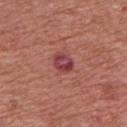Assessment:
Recorded during total-body skin imaging; not selected for excision or biopsy.
Acquisition and patient details:
A roughly 15 mm field-of-view crop from a total-body skin photograph. The patient is a male aged approximately 75. On the chest.